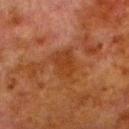Q: Is there a histopathology result?
A: no biopsy performed (imaged during a skin exam)
Q: How large is the lesion?
A: about 4.5 mm
Q: What did automated image analysis measure?
A: border irregularity of about 3.5 on a 0–10 scale and radial color variation of about 0.5; lesion-presence confidence of about 100/100
Q: How was the tile lit?
A: cross-polarized illumination
Q: What kind of image is this?
A: 15 mm crop, total-body photography
Q: Who is the patient?
A: male, approximately 80 years of age
Q: Lesion location?
A: the left lower leg